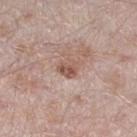Assessment: The lesion was photographed on a routine skin check and not biopsied; there is no pathology result. Context: A 15 mm close-up tile from a total-body photography series done for melanoma screening. On the right thigh. The patient is a male aged approximately 45. Automated image analysis of the tile measured a lesion area of about 4.5 mm², an eccentricity of roughly 0.55, and a symmetry-axis asymmetry near 0.3. The software also gave a border-irregularity rating of about 3/10, a within-lesion color-variation index near 4.5/10, and peripheral color asymmetry of about 2. The recorded lesion diameter is about 2.5 mm. This is a white-light tile.